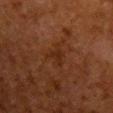Clinical impression: This lesion was catalogued during total-body skin photography and was not selected for biopsy. Background: Imaged with cross-polarized lighting. Approximately 3 mm at its widest. A region of skin cropped from a whole-body photographic capture, roughly 15 mm wide. Located on the front of the torso. The patient is a female approximately 50 years of age.